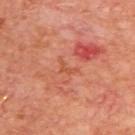The lesion was tiled from a total-body skin photograph and was not biopsied. The lesion is located on the upper back. Captured under cross-polarized illumination. The total-body-photography lesion software estimated an automated nevus-likeness rating near 0 out of 100 and a detector confidence of about 90 out of 100 that the crop contains a lesion. Longest diameter approximately 3 mm. A lesion tile, about 15 mm wide, cut from a 3D total-body photograph. A male subject in their 70s.